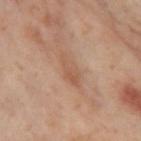biopsy status = total-body-photography surveillance lesion; no biopsy
location = the left thigh
acquisition = ~15 mm crop, total-body skin-cancer survey
subject = female, in their mid- to late 50s
tile lighting = cross-polarized illumination